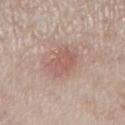Image and clinical context: Located on the right lower leg. Longest diameter approximately 4 mm. This is a white-light tile. A female subject, aged 58 to 62. This image is a 15 mm lesion crop taken from a total-body photograph.